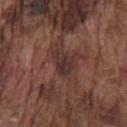Assessment: This lesion was catalogued during total-body skin photography and was not selected for biopsy. Acquisition and patient details: Cropped from a whole-body photographic skin survey; the tile spans about 15 mm. Automated tile analysis of the lesion measured a lesion area of about 5.5 mm², an outline eccentricity of about 0.7 (0 = round, 1 = elongated), and a symmetry-axis asymmetry near 0.5. The software also gave an average lesion color of about L≈31 a*≈18 b*≈19 (CIELAB), about 8 CIELAB-L* units darker than the surrounding skin, and a normalized lesion–skin contrast near 8.5. The software also gave a border-irregularity rating of about 5/10, a color-variation rating of about 2.5/10, and a peripheral color-asymmetry measure near 1. A male patient, aged 73–77. The tile uses white-light illumination. The lesion is on the chest.